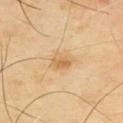workup: catalogued during a skin exam; not biopsied | image-analysis metrics: a border-irregularity rating of about 2.5/10, internal color variation of about 4 on a 0–10 scale, and radial color variation of about 1.5 | subject: male, in their mid-60s | image: ~15 mm crop, total-body skin-cancer survey | anatomic site: the upper back | illumination: cross-polarized illumination.